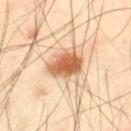Q: Was this lesion biopsied?
A: total-body-photography surveillance lesion; no biopsy
Q: What are the patient's age and sex?
A: male, in their 40s
Q: Illumination type?
A: cross-polarized illumination
Q: What is the imaging modality?
A: ~15 mm tile from a whole-body skin photo
Q: Lesion location?
A: the left thigh
Q: Automated lesion metrics?
A: an area of roughly 10 mm², a shape eccentricity near 0.55, and two-axis asymmetry of about 0.2; a lesion color around L≈63 a*≈24 b*≈40 in CIELAB and a normalized lesion–skin contrast near 10.5; a classifier nevus-likeness of about 100/100 and a detector confidence of about 100 out of 100 that the crop contains a lesion